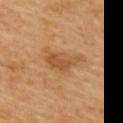| feature | finding |
|---|---|
| workup | total-body-photography surveillance lesion; no biopsy |
| subject | female, aged 58 to 62 |
| site | the upper back |
| illumination | cross-polarized |
| lesion size | about 4.5 mm |
| image source | 15 mm crop, total-body photography |